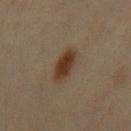Findings:
* workup — catalogued during a skin exam; not biopsied
* image — ~15 mm crop, total-body skin-cancer survey
* subject — male, in their 60s
* site — the chest
* image-analysis metrics — a lesion color around L≈32 a*≈15 b*≈26 in CIELAB and a lesion–skin lightness drop of about 10; a nevus-likeness score of about 100/100 and a detector confidence of about 100 out of 100 that the crop contains a lesion
* tile lighting — cross-polarized illumination
* lesion size — ≈4 mm